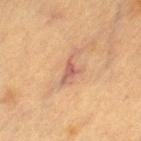A 15 mm crop from a total-body photograph taken for skin-cancer surveillance. The lesion is on the left thigh. A female subject, aged approximately 55. The total-body-photography lesion software estimated an automated nevus-likeness rating near 0 out of 100. Longest diameter approximately 4.5 mm.